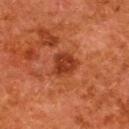Imaged during a routine full-body skin examination; the lesion was not biopsied and no histopathology is available. On the upper back. Automated image analysis of the tile measured a lesion-to-skin contrast of about 8.5 (normalized; higher = more distinct). It also reported a nevus-likeness score of about 80/100 and a lesion-detection confidence of about 100/100. A female patient, about 50 years old. Measured at roughly 3.5 mm in maximum diameter. A lesion tile, about 15 mm wide, cut from a 3D total-body photograph.